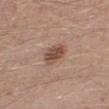Impression:
The lesion was tiled from a total-body skin photograph and was not biopsied.
Background:
The lesion-visualizer software estimated a lesion color around L≈49 a*≈20 b*≈26 in CIELAB, about 12 CIELAB-L* units darker than the surrounding skin, and a normalized lesion–skin contrast near 8.5. A male subject, in their 30s. Measured at roughly 3 mm in maximum diameter. The lesion is located on the left lower leg. Cropped from a whole-body photographic skin survey; the tile spans about 15 mm. The tile uses white-light illumination.